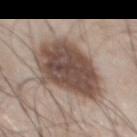Q: Illumination type?
A: white-light
Q: Who is the patient?
A: male, in their mid- to late 50s
Q: What is the imaging modality?
A: 15 mm crop, total-body photography
Q: Where on the body is the lesion?
A: the chest
Q: Lesion size?
A: about 7.5 mm